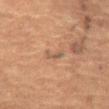Assessment:
The lesion was tiled from a total-body skin photograph and was not biopsied.
Context:
The lesion-visualizer software estimated a footprint of about 2.5 mm², an outline eccentricity of about 0.95 (0 = round, 1 = elongated), and a symmetry-axis asymmetry near 0.35. And it measured roughly 6 lightness units darker than nearby skin and a normalized lesion–skin contrast near 5.5. A female patient roughly 70 years of age. Captured under cross-polarized illumination. A region of skin cropped from a whole-body photographic capture, roughly 15 mm wide. On the front of the torso.